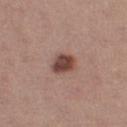Q: Is there a histopathology result?
A: imaged on a skin check; not biopsied
Q: Where on the body is the lesion?
A: the left thigh
Q: How was this image acquired?
A: total-body-photography crop, ~15 mm field of view
Q: Automated lesion metrics?
A: a within-lesion color-variation index near 4.5/10 and peripheral color asymmetry of about 1.5
Q: Who is the patient?
A: female, about 20 years old
Q: Illumination type?
A: white-light